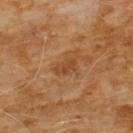Captured during whole-body skin photography for melanoma surveillance; the lesion was not biopsied.
On the chest.
A male patient, aged around 60.
Cropped from a total-body skin-imaging series; the visible field is about 15 mm.
Measured at roughly 3 mm in maximum diameter.
This is a cross-polarized tile.
The lesion-visualizer software estimated an average lesion color of about L≈43 a*≈23 b*≈35 (CIELAB) and a lesion-to-skin contrast of about 6 (normalized; higher = more distinct). It also reported border irregularity of about 3 on a 0–10 scale and a color-variation rating of about 1.5/10.